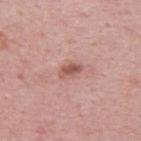  biopsy_status: not biopsied; imaged during a skin examination
  image:
    source: total-body photography crop
    field_of_view_mm: 15
  patient:
    sex: male
    age_approx: 50
  site: upper back
  lighting: white-light
  lesion_size:
    long_diameter_mm_approx: 2.5